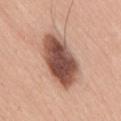workup: total-body-photography surveillance lesion; no biopsy | site: the lower back | size: about 7 mm | patient: male, approximately 55 years of age | lighting: white-light illumination | acquisition: total-body-photography crop, ~15 mm field of view | image-analysis metrics: an area of roughly 23 mm², an eccentricity of roughly 0.85, and two-axis asymmetry of about 0.15; a border-irregularity index near 1.5/10 and a within-lesion color-variation index near 7.5/10; a nevus-likeness score of about 45/100.